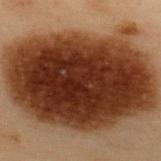Clinical impression:
Recorded during total-body skin imaging; not selected for excision or biopsy.
Clinical summary:
A 15 mm crop from a total-body photograph taken for skin-cancer surveillance. On the upper back. A male subject, approximately 55 years of age.